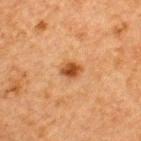<tbp_lesion>
  <biopsy_status>not biopsied; imaged during a skin examination</biopsy_status>
  <image>
    <source>total-body photography crop</source>
    <field_of_view_mm>15</field_of_view_mm>
  </image>
  <automated_metrics>
    <vs_skin_darker_L>11.0</vs_skin_darker_L>
    <vs_skin_contrast_norm>9.5</vs_skin_contrast_norm>
    <border_irregularity_0_10>1.5</border_irregularity_0_10>
    <color_variation_0_10>4.0</color_variation_0_10>
    <peripheral_color_asymmetry>1.0</peripheral_color_asymmetry>
  </automated_metrics>
  <patient>
    <sex>male</sex>
    <age_approx>50</age_approx>
  </patient>
  <site>back</site>
  <lighting>cross-polarized</lighting>
</tbp_lesion>